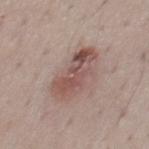Impression:
No biopsy was performed on this lesion — it was imaged during a full skin examination and was not determined to be concerning.
Acquisition and patient details:
Longest diameter approximately 6 mm. The lesion is on the mid back. This is a white-light tile. A male subject aged 48 to 52. A 15 mm close-up extracted from a 3D total-body photography capture.